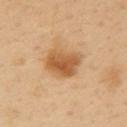Q: Was a biopsy performed?
A: catalogued during a skin exam; not biopsied
Q: What is the lesion's diameter?
A: about 4 mm
Q: Where on the body is the lesion?
A: the right upper arm
Q: How was this image acquired?
A: 15 mm crop, total-body photography
Q: How was the tile lit?
A: cross-polarized illumination
Q: Automated lesion metrics?
A: a color-variation rating of about 4.5/10 and radial color variation of about 1.5
Q: Who is the patient?
A: male, aged 38–42